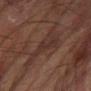Clinical impression:
Imaged during a routine full-body skin examination; the lesion was not biopsied and no histopathology is available.
Background:
From the left forearm. Captured under cross-polarized illumination. An algorithmic analysis of the crop reported an outline eccentricity of about 0.65 (0 = round, 1 = elongated). The analysis additionally found a within-lesion color-variation index near 2.5/10 and radial color variation of about 1. The patient is a male aged approximately 65. A lesion tile, about 15 mm wide, cut from a 3D total-body photograph.